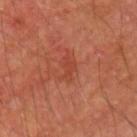- follow-up: no biopsy performed (imaged during a skin exam)
- body site: the left upper arm
- image-analysis metrics: a mean CIELAB color near L≈39 a*≈28 b*≈30 and a normalized border contrast of about 4.5; a border-irregularity rating of about 4.5/10; a classifier nevus-likeness of about 0/100 and a detector confidence of about 100 out of 100 that the crop contains a lesion
- lighting: cross-polarized illumination
- patient: male, aged approximately 65
- image: total-body-photography crop, ~15 mm field of view
- lesion size: ≈3.5 mm